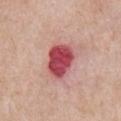{
  "patient": {
    "sex": "male",
    "age_approx": 60
  },
  "site": "chest",
  "lighting": "white-light",
  "image": {
    "source": "total-body photography crop",
    "field_of_view_mm": 15
  },
  "lesion_size": {
    "long_diameter_mm_approx": 4.0
  }
}